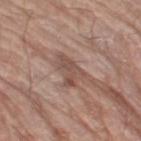Cropped from a whole-body photographic skin survey; the tile spans about 15 mm. On the leg. The tile uses white-light illumination. About 4 mm across. The patient is a male aged 78–82.